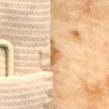Q: Was a biopsy performed?
A: catalogued during a skin exam; not biopsied
Q: Lesion location?
A: the mid back
Q: Patient demographics?
A: female, roughly 60 years of age
Q: How was this image acquired?
A: total-body-photography crop, ~15 mm field of view